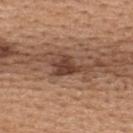This lesion was catalogued during total-body skin photography and was not selected for biopsy.
On the upper back.
A female patient roughly 45 years of age.
A 15 mm close-up extracted from a 3D total-body photography capture.
Imaged with white-light lighting.
Longest diameter approximately 4.5 mm.